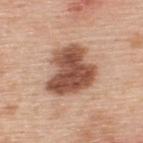Clinical impression:
Captured during whole-body skin photography for melanoma surveillance; the lesion was not biopsied.
Image and clinical context:
A male patient, aged 48–52. The total-body-photography lesion software estimated a shape eccentricity near 0.55 and a shape-asymmetry score of about 0.35 (0 = symmetric). It also reported a mean CIELAB color near L≈52 a*≈22 b*≈30, a lesion–skin lightness drop of about 17, and a lesion-to-skin contrast of about 11.5 (normalized; higher = more distinct). A 15 mm close-up extracted from a 3D total-body photography capture. Located on the upper back. The tile uses white-light illumination.